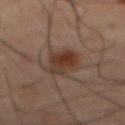<lesion>
<biopsy_status>not biopsied; imaged during a skin examination</biopsy_status>
<automated_metrics>
  <area_mm2_approx>8.0</area_mm2_approx>
  <shape_asymmetry>0.25</shape_asymmetry>
  <cielab_L>25</cielab_L>
  <cielab_a>14</cielab_a>
  <cielab_b>20</cielab_b>
  <vs_skin_darker_L>8.0</vs_skin_darker_L>
  <vs_skin_contrast_norm>9.0</vs_skin_contrast_norm>
  <nevus_likeness_0_100>85</nevus_likeness_0_100>
</automated_metrics>
<site>abdomen</site>
<lighting>cross-polarized</lighting>
<patient>
  <sex>male</sex>
  <age_approx>60</age_approx>
</patient>
<lesion_size>
  <long_diameter_mm_approx>4.5</long_diameter_mm_approx>
</lesion_size>
<image>
  <source>total-body photography crop</source>
  <field_of_view_mm>15</field_of_view_mm>
</image>
</lesion>